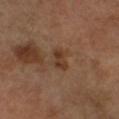Assessment:
The lesion was tiled from a total-body skin photograph and was not biopsied.
Context:
On the right lower leg. The total-body-photography lesion software estimated an area of roughly 4.5 mm², a shape eccentricity near 0.8, and a symmetry-axis asymmetry near 0.3. The software also gave an average lesion color of about L≈36 a*≈18 b*≈29 (CIELAB), about 8 CIELAB-L* units darker than the surrounding skin, and a normalized lesion–skin contrast near 7. And it measured a classifier nevus-likeness of about 5/100 and a lesion-detection confidence of about 100/100. A male subject roughly 65 years of age. A region of skin cropped from a whole-body photographic capture, roughly 15 mm wide.